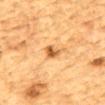Context: A male patient aged 83 or older. On the mid back. This image is a 15 mm lesion crop taken from a total-body photograph.